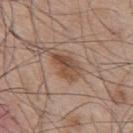Imaged during a routine full-body skin examination; the lesion was not biopsied and no histopathology is available.
The lesion's longest dimension is about 4 mm.
Cropped from a whole-body photographic skin survey; the tile spans about 15 mm.
On the mid back.
A male subject aged approximately 55.